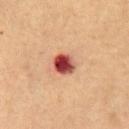No biopsy was performed on this lesion — it was imaged during a full skin examination and was not determined to be concerning.
The tile uses cross-polarized illumination.
A male patient, approximately 65 years of age.
The recorded lesion diameter is about 2.5 mm.
An algorithmic analysis of the crop reported a mean CIELAB color near L≈44 a*≈32 b*≈28, a lesion–skin lightness drop of about 20, and a normalized lesion–skin contrast near 14.5. The software also gave a classifier nevus-likeness of about 0/100.
A 15 mm crop from a total-body photograph taken for skin-cancer surveillance.
Located on the mid back.